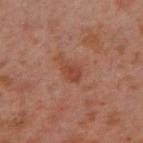Part of a total-body skin-imaging series; this lesion was reviewed on a skin check and was not flagged for biopsy.
The lesion is on the right upper arm.
The recorded lesion diameter is about 4 mm.
A male subject, in their 30s.
This image is a 15 mm lesion crop taken from a total-body photograph.
Imaged with cross-polarized lighting.